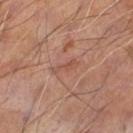Q: Was this lesion biopsied?
A: total-body-photography surveillance lesion; no biopsy
Q: What are the patient's age and sex?
A: male, aged 53 to 57
Q: What kind of image is this?
A: ~15 mm tile from a whole-body skin photo
Q: Lesion location?
A: the left thigh